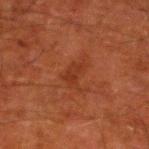<record>
  <automated_metrics>
    <vs_skin_darker_L>5.0</vs_skin_darker_L>
    <vs_skin_contrast_norm>5.0</vs_skin_contrast_norm>
    <nevus_likeness_0_100>10</nevus_likeness_0_100>
    <lesion_detection_confidence_0_100>100</lesion_detection_confidence_0_100>
  </automated_metrics>
  <patient>
    <sex>male</sex>
    <age_approx>80</age_approx>
  </patient>
  <site>left lower leg</site>
  <lesion_size>
    <long_diameter_mm_approx>3.0</long_diameter_mm_approx>
  </lesion_size>
  <image>
    <source>total-body photography crop</source>
    <field_of_view_mm>15</field_of_view_mm>
  </image>
</record>